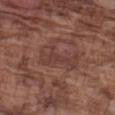Captured during whole-body skin photography for melanoma surveillance; the lesion was not biopsied. Automated image analysis of the tile measured an outline eccentricity of about 0.9 (0 = round, 1 = elongated). And it measured a lesion-detection confidence of about 80/100. Cropped from a total-body skin-imaging series; the visible field is about 15 mm. On the arm. The subject is a male aged approximately 75. The tile uses white-light illumination.